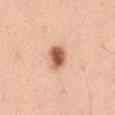The lesion was photographed on a routine skin check and not biopsied; there is no pathology result.
A male subject aged 48 to 52.
Located on the abdomen.
The lesion's longest dimension is about 3 mm.
A 15 mm close-up extracted from a 3D total-body photography capture.